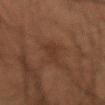{
  "biopsy_status": "not biopsied; imaged during a skin examination",
  "lesion_size": {
    "long_diameter_mm_approx": 2.5
  },
  "site": "right forearm",
  "patient": {
    "sex": "male",
    "age_approx": 55
  },
  "image": {
    "source": "total-body photography crop",
    "field_of_view_mm": 15
  },
  "automated_metrics": {
    "area_mm2_approx": 3.0,
    "eccentricity": 0.8,
    "shape_asymmetry": 0.25,
    "nevus_likeness_0_100": 0,
    "lesion_detection_confidence_0_100": 90
  },
  "lighting": "cross-polarized"
}